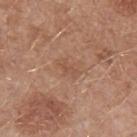<record>
<biopsy_status>not biopsied; imaged during a skin examination</biopsy_status>
<lighting>white-light</lighting>
<patient>
  <sex>male</sex>
  <age_approx>55</age_approx>
</patient>
<image>
  <source>total-body photography crop</source>
  <field_of_view_mm>15</field_of_view_mm>
</image>
<site>left upper arm</site>
<automated_metrics>
  <nevus_likeness_0_100>0</nevus_likeness_0_100>
  <lesion_detection_confidence_0_100>100</lesion_detection_confidence_0_100>
</automated_metrics>
<lesion_size>
  <long_diameter_mm_approx>2.5</long_diameter_mm_approx>
</lesion_size>
</record>